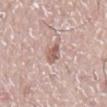notes: no biopsy performed (imaged during a skin exam)
image-analysis metrics: an area of roughly 4 mm² and an eccentricity of roughly 0.9; border irregularity of about 4 on a 0–10 scale and a color-variation rating of about 1.5/10; a classifier nevus-likeness of about 5/100 and a lesion-detection confidence of about 90/100
imaging modality: ~15 mm crop, total-body skin-cancer survey
patient: male, aged approximately 75
site: the mid back